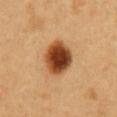workup = total-body-photography surveillance lesion; no biopsy | patient = male, aged around 50 | anatomic site = the front of the torso | illumination = cross-polarized | acquisition = 15 mm crop, total-body photography | diameter = ≈5 mm.